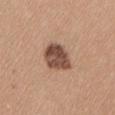Findings:
- notes — imaged on a skin check; not biopsied
- acquisition — ~15 mm tile from a whole-body skin photo
- illumination — white-light illumination
- site — the left thigh
- subject — female, in their 40s